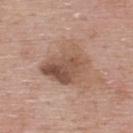notes = total-body-photography surveillance lesion; no biopsy
diameter = ≈6 mm
site = the upper back
automated lesion analysis = a lesion area of about 18 mm² and a symmetry-axis asymmetry near 0.25; an average lesion color of about L≈52 a*≈19 b*≈27 (CIELAB) and roughly 11 lightness units darker than nearby skin; a border-irregularity rating of about 3/10, a within-lesion color-variation index near 7.5/10, and peripheral color asymmetry of about 3
tile lighting = white-light
patient = male, in their mid-50s
imaging modality = ~15 mm tile from a whole-body skin photo